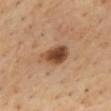<lesion>
<biopsy_status>not biopsied; imaged during a skin examination</biopsy_status>
<site>back</site>
<lighting>cross-polarized</lighting>
<image>
  <source>total-body photography crop</source>
  <field_of_view_mm>15</field_of_view_mm>
</image>
<lesion_size>
  <long_diameter_mm_approx>4.0</long_diameter_mm_approx>
</lesion_size>
<patient>
  <sex>male</sex>
  <age_approx>55</age_approx>
</patient>
<automated_metrics>
  <nevus_likeness_0_100>95</nevus_likeness_0_100>
  <lesion_detection_confidence_0_100>100</lesion_detection_confidence_0_100>
</automated_metrics>
</lesion>